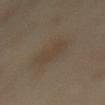Part of a total-body skin-imaging series; this lesion was reviewed on a skin check and was not flagged for biopsy. The patient is a female roughly 55 years of age. Captured under cross-polarized illumination. A 15 mm close-up extracted from a 3D total-body photography capture. The lesion's longest dimension is about 4 mm. An algorithmic analysis of the crop reported a shape eccentricity near 0.35 and a symmetry-axis asymmetry near 0.2. It also reported a border-irregularity rating of about 2.5/10 and a color-variation rating of about 1.5/10. From the back.